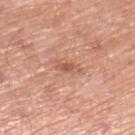The lesion was tiled from a total-body skin photograph and was not biopsied. A close-up tile cropped from a whole-body skin photograph, about 15 mm across. The recorded lesion diameter is about 3.5 mm. Captured under white-light illumination. Automated tile analysis of the lesion measured a lesion area of about 4 mm², a shape eccentricity near 0.9, and two-axis asymmetry of about 0.35. The analysis additionally found a mean CIELAB color near L≈58 a*≈24 b*≈31, roughly 9 lightness units darker than nearby skin, and a lesion-to-skin contrast of about 6 (normalized; higher = more distinct). From the right lower leg. The patient is a male aged around 80.